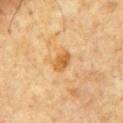The lesion was tiled from a total-body skin photograph and was not biopsied.
A male patient, about 65 years old.
The lesion is located on the chest.
A roughly 15 mm field-of-view crop from a total-body skin photograph.
Imaged with cross-polarized lighting.